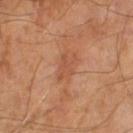Q: How was this image acquired?
A: ~15 mm crop, total-body skin-cancer survey
Q: What are the patient's age and sex?
A: male, aged 63 to 67
Q: What lighting was used for the tile?
A: cross-polarized illumination